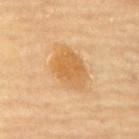Q: Was a biopsy performed?
A: catalogued during a skin exam; not biopsied
Q: Patient demographics?
A: male, in their mid-80s or older
Q: Lesion size?
A: ≈5.5 mm
Q: What kind of image is this?
A: 15 mm crop, total-body photography
Q: How was the tile lit?
A: cross-polarized
Q: What is the anatomic site?
A: the mid back